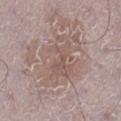Assessment: This lesion was catalogued during total-body skin photography and was not selected for biopsy. Background: Located on the right lower leg. A lesion tile, about 15 mm wide, cut from a 3D total-body photograph. The tile uses white-light illumination. The recorded lesion diameter is about 6.5 mm. A male patient aged around 70. Automated tile analysis of the lesion measured a footprint of about 27 mm², an outline eccentricity of about 0.65 (0 = round, 1 = elongated), and two-axis asymmetry of about 0.25. The software also gave a mean CIELAB color near L≈56 a*≈15 b*≈21, about 7 CIELAB-L* units darker than the surrounding skin, and a normalized border contrast of about 5. The analysis additionally found a border-irregularity index near 4/10, internal color variation of about 4.5 on a 0–10 scale, and radial color variation of about 1.5.